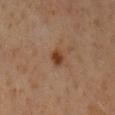Captured during whole-body skin photography for melanoma surveillance; the lesion was not biopsied. A close-up tile cropped from a whole-body skin photograph, about 15 mm across. The subject is a male aged 58 to 62. On the mid back.